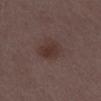Recorded during total-body skin imaging; not selected for excision or biopsy. An algorithmic analysis of the crop reported a footprint of about 7 mm², an eccentricity of roughly 0.65, and a symmetry-axis asymmetry near 0.15. The software also gave a border-irregularity rating of about 1.5/10 and a peripheral color-asymmetry measure near 1. Cropped from a whole-body photographic skin survey; the tile spans about 15 mm. This is a white-light tile. A female subject aged approximately 30. Located on the right thigh.